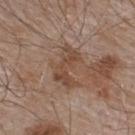Recorded during total-body skin imaging; not selected for excision or biopsy.
The patient is a male aged approximately 55.
The tile uses white-light illumination.
A 15 mm close-up tile from a total-body photography series done for melanoma screening.
Longest diameter approximately 5 mm.
The lesion is located on the upper back.